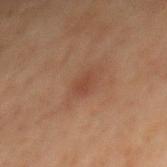Impression: No biopsy was performed on this lesion — it was imaged during a full skin examination and was not determined to be concerning. Background: The total-body-photography lesion software estimated an area of roughly 3 mm², a shape eccentricity near 0.8, and a symmetry-axis asymmetry near 0.2. And it measured an average lesion color of about L≈33 a*≈18 b*≈24 (CIELAB), about 5 CIELAB-L* units darker than the surrounding skin, and a normalized border contrast of about 5.5. The software also gave a border-irregularity index near 2/10 and peripheral color asymmetry of about 0.5. Located on the mid back. Measured at roughly 2.5 mm in maximum diameter. Captured under cross-polarized illumination. The patient is a male aged 68–72. This image is a 15 mm lesion crop taken from a total-body photograph.